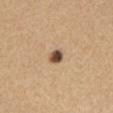workup: catalogued during a skin exam; not biopsied
anatomic site: the front of the torso
acquisition: 15 mm crop, total-body photography
automated lesion analysis: a lesion area of about 3.5 mm², a shape eccentricity near 0.45, and a shape-asymmetry score of about 0.25 (0 = symmetric); a border-irregularity rating of about 2/10 and peripheral color asymmetry of about 1
lesion size: ~2 mm (longest diameter)
patient: female, aged approximately 60